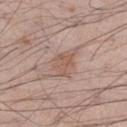The lesion was tiled from a total-body skin photograph and was not biopsied. On the right thigh. Cropped from a total-body skin-imaging series; the visible field is about 15 mm. Measured at roughly 3 mm in maximum diameter. A male patient, approximately 55 years of age. Imaged with white-light lighting. An algorithmic analysis of the crop reported a lesion–skin lightness drop of about 7 and a normalized border contrast of about 5.5. And it measured a within-lesion color-variation index near 2/10 and peripheral color asymmetry of about 0.5. The software also gave lesion-presence confidence of about 100/100.